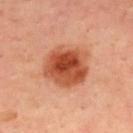<tbp_lesion>
  <biopsy_status>not biopsied; imaged during a skin examination</biopsy_status>
  <patient>
    <sex>male</sex>
    <age_approx>50</age_approx>
  </patient>
  <automated_metrics>
    <area_mm2_approx>20.0</area_mm2_approx>
    <eccentricity>0.55</eccentricity>
    <cielab_L>51</cielab_L>
    <cielab_a>30</cielab_a>
    <cielab_b>37</cielab_b>
    <vs_skin_darker_L>15.0</vs_skin_darker_L>
    <vs_skin_contrast_norm>10.5</vs_skin_contrast_norm>
  </automated_metrics>
  <image>
    <source>total-body photography crop</source>
    <field_of_view_mm>15</field_of_view_mm>
  </image>
  <lighting>cross-polarized</lighting>
  <site>upper back</site>
</tbp_lesion>